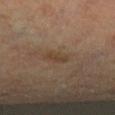{
  "biopsy_status": "not biopsied; imaged during a skin examination",
  "patient": {
    "sex": "female",
    "age_approx": 65
  },
  "image": {
    "source": "total-body photography crop",
    "field_of_view_mm": 15
  },
  "site": "leg",
  "lighting": "cross-polarized",
  "automated_metrics": {
    "cielab_L": 39,
    "cielab_a": 14,
    "cielab_b": 27,
    "vs_skin_darker_L": 6.0,
    "vs_skin_contrast_norm": 6.0,
    "lesion_detection_confidence_0_100": 100
  },
  "lesion_size": {
    "long_diameter_mm_approx": 2.5
  }
}